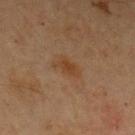follow-up = catalogued during a skin exam; not biopsied
image = 15 mm crop, total-body photography
location = the arm
lighting = cross-polarized illumination
automated metrics = a footprint of about 4.5 mm²; an automated nevus-likeness rating near 50 out of 100 and a lesion-detection confidence of about 100/100
patient = female, aged 68 to 72
lesion size = ~3.5 mm (longest diameter)